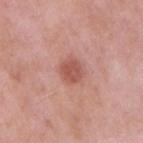biopsy status: no biopsy performed (imaged during a skin exam) | imaging modality: ~15 mm tile from a whole-body skin photo | patient: male, aged approximately 55 | image-analysis metrics: border irregularity of about 1.5 on a 0–10 scale and a color-variation rating of about 2.5/10 | site: the right upper arm | tile lighting: white-light illumination | lesion diameter: about 3 mm.